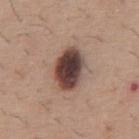Recorded during total-body skin imaging; not selected for excision or biopsy. Cropped from a whole-body photographic skin survey; the tile spans about 15 mm. Captured under white-light illumination. The lesion's longest dimension is about 5 mm. The lesion is located on the abdomen. A male subject about 60 years old.